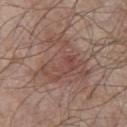* patient · male, aged approximately 80
* size · ~7 mm (longest diameter)
* acquisition · total-body-photography crop, ~15 mm field of view
* automated metrics · an area of roughly 22 mm² and an outline eccentricity of about 0.45 (0 = round, 1 = elongated); a lesion color around L≈48 a*≈19 b*≈23 in CIELAB and a normalized lesion–skin contrast near 5.5; a border-irregularity index near 7/10, a within-lesion color-variation index near 4.5/10, and a peripheral color-asymmetry measure near 1.5; an automated nevus-likeness rating near 0 out of 100 and a detector confidence of about 100 out of 100 that the crop contains a lesion
* anatomic site · the chest
* lighting · white-light